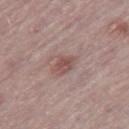<lesion>
  <site>right thigh</site>
  <patient>
    <sex>male</sex>
    <age_approx>75</age_approx>
  </patient>
  <image>
    <source>total-body photography crop</source>
    <field_of_view_mm>15</field_of_view_mm>
  </image>
  <lesion_size>
    <long_diameter_mm_approx>3.0</long_diameter_mm_approx>
  </lesion_size>
  <lighting>white-light</lighting>
</lesion>